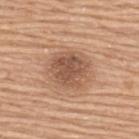Case summary:
- biopsy status — imaged on a skin check; not biopsied
- body site — the back
- lesion size — ≈4 mm
- patient — female, aged around 60
- illumination — white-light
- image source — total-body-photography crop, ~15 mm field of view